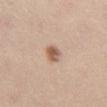Findings:
* notes · total-body-photography surveillance lesion; no biopsy
* tile lighting · white-light illumination
* size · about 3 mm
* automated lesion analysis · a footprint of about 4 mm² and two-axis asymmetry of about 0.15; a border-irregularity rating of about 1.5/10, a within-lesion color-variation index near 3.5/10, and peripheral color asymmetry of about 1; a nevus-likeness score of about 90/100 and lesion-presence confidence of about 100/100
* subject · female, aged 38 to 42
* image · ~15 mm crop, total-body skin-cancer survey
* site · the right thigh